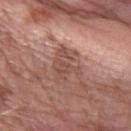Impression:
This lesion was catalogued during total-body skin photography and was not selected for biopsy.
Acquisition and patient details:
A roughly 15 mm field-of-view crop from a total-body skin photograph. An algorithmic analysis of the crop reported an area of roughly 9.5 mm², a shape eccentricity near 0.65, and a shape-asymmetry score of about 0.45 (0 = symmetric). And it measured a lesion–skin lightness drop of about 9 and a normalized lesion–skin contrast near 6.5. The subject is a female roughly 60 years of age. About 4.5 mm across. The tile uses white-light illumination. The lesion is on the right forearm.